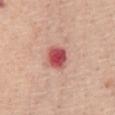Q: What kind of image is this?
A: ~15 mm crop, total-body skin-cancer survey
Q: What did automated image analysis measure?
A: a mean CIELAB color near L≈53 a*≈35 b*≈25, about 16 CIELAB-L* units darker than the surrounding skin, and a normalized border contrast of about 10.5; a nevus-likeness score of about 0/100 and a detector confidence of about 100 out of 100 that the crop contains a lesion
Q: Who is the patient?
A: female, about 55 years old
Q: How was the tile lit?
A: white-light illumination
Q: What is the lesion's diameter?
A: ~3 mm (longest diameter)
Q: What is the anatomic site?
A: the abdomen